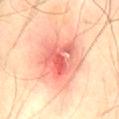This lesion was catalogued during total-body skin photography and was not selected for biopsy. Located on the lower back. A close-up tile cropped from a whole-body skin photograph, about 15 mm across. A male patient, approximately 45 years of age. Longest diameter approximately 8 mm.